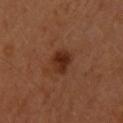Notes:
– notes · no biopsy performed (imaged during a skin exam)
– subject · female, about 35 years old
– site · the upper back
– imaging modality · total-body-photography crop, ~15 mm field of view